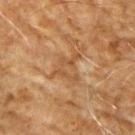Q: Patient demographics?
A: male, aged around 70
Q: Automated lesion metrics?
A: a mean CIELAB color near L≈42 a*≈18 b*≈32, roughly 6 lightness units darker than nearby skin, and a normalized border contrast of about 5.5; a color-variation rating of about 0/10; a nevus-likeness score of about 0/100 and a lesion-detection confidence of about 55/100
Q: What lighting was used for the tile?
A: cross-polarized
Q: How was this image acquired?
A: ~15 mm tile from a whole-body skin photo
Q: What is the lesion's diameter?
A: about 4 mm
Q: Where on the body is the lesion?
A: the left upper arm